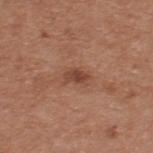notes — catalogued during a skin exam; not biopsied
illumination — white-light illumination
patient — male, aged approximately 55
imaging modality — 15 mm crop, total-body photography
TBP lesion metrics — an area of roughly 4 mm², an outline eccentricity of about 0.8 (0 = round, 1 = elongated), and two-axis asymmetry of about 0.25; border irregularity of about 2.5 on a 0–10 scale and a color-variation rating of about 2.5/10; a classifier nevus-likeness of about 30/100 and lesion-presence confidence of about 100/100
site — the upper back